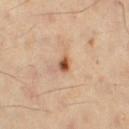| field | value |
|---|---|
| notes | catalogued during a skin exam; not biopsied |
| lesion size | ~2 mm (longest diameter) |
| patient | female, in their mid- to late 40s |
| image | total-body-photography crop, ~15 mm field of view |
| body site | the right thigh |
| tile lighting | cross-polarized illumination |